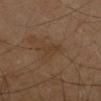Part of a total-body skin-imaging series; this lesion was reviewed on a skin check and was not flagged for biopsy. From the left arm. The total-body-photography lesion software estimated an eccentricity of roughly 0.5. And it measured an average lesion color of about L≈36 a*≈15 b*≈28 (CIELAB) and a normalized lesion–skin contrast near 4.5. It also reported an automated nevus-likeness rating near 0 out of 100. The lesion's longest dimension is about 3 mm. A 15 mm close-up extracted from a 3D total-body photography capture. A male subject, about 50 years old.